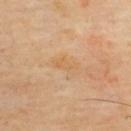Assessment: The lesion was photographed on a routine skin check and not biopsied; there is no pathology result. Acquisition and patient details: The patient is a male aged approximately 65. The recorded lesion diameter is about 3.5 mm. A region of skin cropped from a whole-body photographic capture, roughly 15 mm wide. Imaged with cross-polarized lighting. From the upper back.